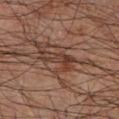{"biopsy_status": "not biopsied; imaged during a skin examination", "lesion_size": {"long_diameter_mm_approx": 4.5}, "image": {"source": "total-body photography crop", "field_of_view_mm": 15}, "site": "right forearm", "lighting": "cross-polarized", "patient": {"sex": "male", "age_approx": 45}}